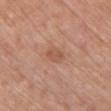{"biopsy_status": "not biopsied; imaged during a skin examination", "image": {"source": "total-body photography crop", "field_of_view_mm": 15}, "patient": {"sex": "female", "age_approx": 65}, "lighting": "white-light", "lesion_size": {"long_diameter_mm_approx": 2.5}, "site": "chest"}